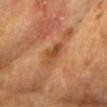workup: total-body-photography surveillance lesion; no biopsy